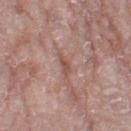workup — catalogued during a skin exam; not biopsied
size — ~3 mm (longest diameter)
imaging modality — total-body-photography crop, ~15 mm field of view
image-analysis metrics — a footprint of about 3 mm², an outline eccentricity of about 0.9 (0 = round, 1 = elongated), and a symmetry-axis asymmetry near 0.4; an automated nevus-likeness rating near 0 out of 100
patient — female, roughly 75 years of age
anatomic site — the left thigh
illumination — white-light illumination